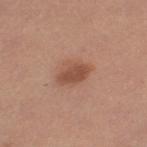Recorded during total-body skin imaging; not selected for excision or biopsy.
Measured at roughly 4 mm in maximum diameter.
A 15 mm close-up tile from a total-body photography series done for melanoma screening.
The lesion is on the left thigh.
A female subject aged approximately 35.
The tile uses white-light illumination.